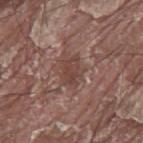This lesion was catalogued during total-body skin photography and was not selected for biopsy. The lesion is located on the mid back. An algorithmic analysis of the crop reported an eccentricity of roughly 0.7 and a symmetry-axis asymmetry near 0.25. The software also gave a mean CIELAB color near L≈43 a*≈19 b*≈23 and a lesion–skin lightness drop of about 8. This image is a 15 mm lesion crop taken from a total-body photograph. A male subject about 40 years old. This is a white-light tile. About 3.5 mm across.